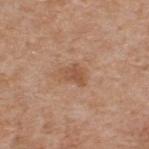Background: The lesion is located on the upper back. Cropped from a total-body skin-imaging series; the visible field is about 15 mm. The subject is a male aged 58–62. Longest diameter approximately 3 mm. This is a white-light tile. The total-body-photography lesion software estimated a shape eccentricity near 0.7. And it measured a classifier nevus-likeness of about 0/100 and a detector confidence of about 100 out of 100 that the crop contains a lesion.